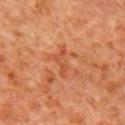Captured during whole-body skin photography for melanoma surveillance; the lesion was not biopsied.
Cropped from a whole-body photographic skin survey; the tile spans about 15 mm.
A male subject, aged approximately 80.
The lesion is on the arm.
This is a cross-polarized tile.
About 3.5 mm across.
The total-body-photography lesion software estimated an area of roughly 3.5 mm² and an eccentricity of roughly 0.9. It also reported a lesion color around L≈40 a*≈24 b*≈32 in CIELAB and a lesion–skin lightness drop of about 6. The software also gave an automated nevus-likeness rating near 0 out of 100.